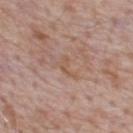Captured during whole-body skin photography for melanoma surveillance; the lesion was not biopsied. A close-up tile cropped from a whole-body skin photograph, about 15 mm across. The lesion-visualizer software estimated a mean CIELAB color near L≈55 a*≈19 b*≈29, about 5 CIELAB-L* units darker than the surrounding skin, and a lesion-to-skin contrast of about 5.5 (normalized; higher = more distinct). On the upper back. The subject is a male aged approximately 65.